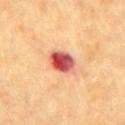image source = ~15 mm crop, total-body skin-cancer survey | automated metrics = a lesion area of about 7.5 mm², an eccentricity of roughly 0.65, and two-axis asymmetry of about 0.15; a lesion color around L≈56 a*≈37 b*≈31 in CIELAB, a lesion–skin lightness drop of about 20, and a normalized border contrast of about 13; a border-irregularity index near 1.5/10 and radial color variation of about 4.5 | subject = male, roughly 85 years of age or older | lesion diameter = ≈3.5 mm | site = the chest | illumination = cross-polarized.